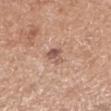The lesion was tiled from a total-body skin photograph and was not biopsied.
Cropped from a total-body skin-imaging series; the visible field is about 15 mm.
Imaged with white-light lighting.
From the right upper arm.
A female patient, approximately 70 years of age.
About 2.5 mm across.